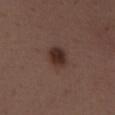Assessment: The lesion was tiled from a total-body skin photograph and was not biopsied. Acquisition and patient details: The recorded lesion diameter is about 3 mm. Located on the chest. A female subject aged 48–52. An algorithmic analysis of the crop reported a footprint of about 6.5 mm² and two-axis asymmetry of about 0.15. The software also gave a mean CIELAB color near L≈30 a*≈17 b*≈21. It also reported border irregularity of about 1.5 on a 0–10 scale, a color-variation rating of about 3.5/10, and peripheral color asymmetry of about 1. The tile uses white-light illumination. A region of skin cropped from a whole-body photographic capture, roughly 15 mm wide.